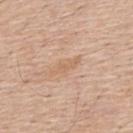follow-up — imaged on a skin check; not biopsied | subject — male, aged around 55 | diameter — ~4 mm (longest diameter) | image source — ~15 mm crop, total-body skin-cancer survey | tile lighting — white-light illumination | body site — the upper back.